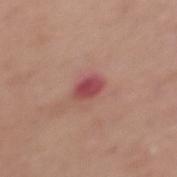Q: Is there a histopathology result?
A: no biopsy performed (imaged during a skin exam)
Q: Where on the body is the lesion?
A: the mid back
Q: Patient demographics?
A: female, aged 63 to 67
Q: What is the lesion's diameter?
A: ~3 mm (longest diameter)
Q: What did automated image analysis measure?
A: a lesion color around L≈47 a*≈31 b*≈22 in CIELAB and a lesion–skin lightness drop of about 12
Q: Illumination type?
A: white-light
Q: How was this image acquired?
A: ~15 mm tile from a whole-body skin photo